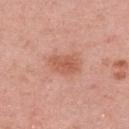Notes:
• workup · imaged on a skin check; not biopsied